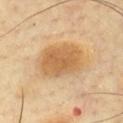notes: total-body-photography surveillance lesion; no biopsy
location: the chest
patient: male, in their mid- to late 50s
lesion diameter: ≈6 mm
tile lighting: cross-polarized illumination
TBP lesion metrics: a lesion area of about 21 mm² and a shape-asymmetry score of about 0.1 (0 = symmetric)
imaging modality: total-body-photography crop, ~15 mm field of view